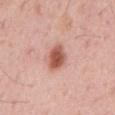Recorded during total-body skin imaging; not selected for excision or biopsy.
Approximately 3 mm at its widest.
Automated tile analysis of the lesion measured an area of roughly 6.5 mm², an eccentricity of roughly 0.55, and two-axis asymmetry of about 0.2. And it measured a border-irregularity rating of about 1.5/10, a within-lesion color-variation index near 4/10, and peripheral color asymmetry of about 1. The analysis additionally found an automated nevus-likeness rating near 100 out of 100 and lesion-presence confidence of about 100/100.
From the mid back.
A male subject, aged 53–57.
Cropped from a total-body skin-imaging series; the visible field is about 15 mm.
Captured under white-light illumination.